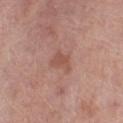Clinical impression: No biopsy was performed on this lesion — it was imaged during a full skin examination and was not determined to be concerning. Acquisition and patient details: A male patient roughly 70 years of age. This image is a 15 mm lesion crop taken from a total-body photograph. The lesion is on the left lower leg.